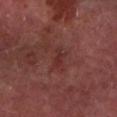Notes:
• subject: male, aged 63 to 67
• automated lesion analysis: about 6 CIELAB-L* units darker than the surrounding skin and a normalized lesion–skin contrast near 5.5; border irregularity of about 4 on a 0–10 scale and peripheral color asymmetry of about 0; a classifier nevus-likeness of about 0/100 and a detector confidence of about 95 out of 100 that the crop contains a lesion
• imaging modality: ~15 mm crop, total-body skin-cancer survey
• location: the left forearm